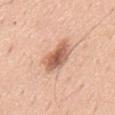Findings:
* follow-up: catalogued during a skin exam; not biopsied
* subject: male, about 40 years old
* image-analysis metrics: a footprint of about 9.5 mm², an eccentricity of roughly 0.75, and a symmetry-axis asymmetry near 0.25; a lesion color around L≈61 a*≈23 b*≈32 in CIELAB, roughly 14 lightness units darker than nearby skin, and a lesion-to-skin contrast of about 8.5 (normalized; higher = more distinct); border irregularity of about 2.5 on a 0–10 scale, a within-lesion color-variation index near 5/10, and a peripheral color-asymmetry measure near 1.5; an automated nevus-likeness rating near 90 out of 100 and lesion-presence confidence of about 100/100
* lesion size: ≈4.5 mm
* image: 15 mm crop, total-body photography
* location: the mid back
* illumination: white-light